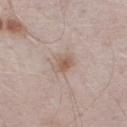<lesion>
<biopsy_status>not biopsied; imaged during a skin examination</biopsy_status>
<lesion_size>
  <long_diameter_mm_approx>3.0</long_diameter_mm_approx>
</lesion_size>
<site>right lower leg</site>
<patient>
  <sex>male</sex>
  <age_approx>40</age_approx>
</patient>
<image>
  <source>total-body photography crop</source>
  <field_of_view_mm>15</field_of_view_mm>
</image>
</lesion>